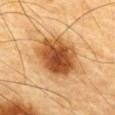Recorded during total-body skin imaging; not selected for excision or biopsy.
Imaged with cross-polarized lighting.
A male subject aged around 85 or older.
The lesion is on the chest.
An algorithmic analysis of the crop reported a mean CIELAB color near L≈45 a*≈22 b*≈37 and a lesion-to-skin contrast of about 11 (normalized; higher = more distinct). The software also gave an automated nevus-likeness rating near 100 out of 100 and a lesion-detection confidence of about 100/100.
This image is a 15 mm lesion crop taken from a total-body photograph.